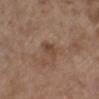| key | value |
|---|---|
| biopsy status | total-body-photography surveillance lesion; no biopsy |
| patient | female, roughly 65 years of age |
| image-analysis metrics | an area of roughly 3.5 mm², an eccentricity of roughly 0.75, and a shape-asymmetry score of about 0.2 (0 = symmetric); an average lesion color of about L≈44 a*≈18 b*≈28 (CIELAB) and a lesion-to-skin contrast of about 6.5 (normalized; higher = more distinct); a nevus-likeness score of about 0/100 and a detector confidence of about 100 out of 100 that the crop contains a lesion |
| image source | ~15 mm crop, total-body skin-cancer survey |
| anatomic site | the left lower leg |
| illumination | white-light illumination |